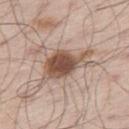Part of a total-body skin-imaging series; this lesion was reviewed on a skin check and was not flagged for biopsy. A male subject in their mid-50s. Located on the right thigh. A region of skin cropped from a whole-body photographic capture, roughly 15 mm wide.